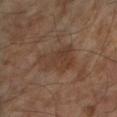No biopsy was performed on this lesion — it was imaged during a full skin examination and was not determined to be concerning. A lesion tile, about 15 mm wide, cut from a 3D total-body photograph. The tile uses cross-polarized illumination. The recorded lesion diameter is about 4 mm. The lesion is on the right forearm. A male patient approximately 65 years of age.